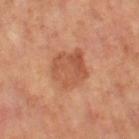Findings:
• tile lighting: cross-polarized
• subject: female, in their mid- to late 60s
• location: the left thigh
• imaging modality: ~15 mm tile from a whole-body skin photo
• automated metrics: a lesion area of about 15 mm², an eccentricity of roughly 0.6, and two-axis asymmetry of about 0.2